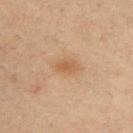biopsy status: imaged on a skin check; not biopsied
imaging modality: 15 mm crop, total-body photography
anatomic site: the left upper arm
subject: male, in their mid-30s